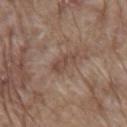Assessment: Captured during whole-body skin photography for melanoma surveillance; the lesion was not biopsied.